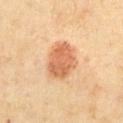Image and clinical context: A male patient, aged 68 to 72. Imaged with cross-polarized lighting. On the chest. Cropped from a total-body skin-imaging series; the visible field is about 15 mm.